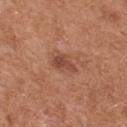Q: Was a biopsy performed?
A: catalogued during a skin exam; not biopsied
Q: What did automated image analysis measure?
A: border irregularity of about 4 on a 0–10 scale and a peripheral color-asymmetry measure near 0.5; a detector confidence of about 100 out of 100 that the crop contains a lesion
Q: Where on the body is the lesion?
A: the upper back
Q: Illumination type?
A: white-light
Q: Lesion size?
A: about 3.5 mm
Q: What kind of image is this?
A: total-body-photography crop, ~15 mm field of view
Q: Patient demographics?
A: female, aged 38–42